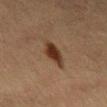Q: Was this lesion biopsied?
A: imaged on a skin check; not biopsied
Q: How large is the lesion?
A: ~4 mm (longest diameter)
Q: Patient demographics?
A: male, aged approximately 65
Q: How was the tile lit?
A: cross-polarized illumination
Q: What did automated image analysis measure?
A: a lesion area of about 6.5 mm², an eccentricity of roughly 0.85, and two-axis asymmetry of about 0.15; a lesion–skin lightness drop of about 11 and a lesion-to-skin contrast of about 11 (normalized; higher = more distinct); a classifier nevus-likeness of about 100/100
Q: What is the imaging modality?
A: ~15 mm crop, total-body skin-cancer survey
Q: Where on the body is the lesion?
A: the abdomen